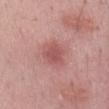{"biopsy_status": "not biopsied; imaged during a skin examination", "lighting": "white-light", "site": "back", "image": {"source": "total-body photography crop", "field_of_view_mm": 15}, "patient": {"sex": "male", "age_approx": 65}, "automated_metrics": {"cielab_L": 53, "cielab_a": 27, "cielab_b": 23, "vs_skin_darker_L": 9.0, "border_irregularity_0_10": 2.5, "color_variation_0_10": 2.0, "nevus_likeness_0_100": 20}, "lesion_size": {"long_diameter_mm_approx": 3.0}}